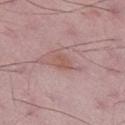<case>
<patient>
  <sex>male</sex>
  <age_approx>50</age_approx>
</patient>
<lesion_size>
  <long_diameter_mm_approx>2.5</long_diameter_mm_approx>
</lesion_size>
<site>right lower leg</site>
<image>
  <source>total-body photography crop</source>
  <field_of_view_mm>15</field_of_view_mm>
</image>
<automated_metrics>
  <eccentricity>0.7</eccentricity>
  <shape_asymmetry>0.25</shape_asymmetry>
  <border_irregularity_0_10>2.5</border_irregularity_0_10>
  <color_variation_0_10>1.5</color_variation_0_10>
  <peripheral_color_asymmetry>0.5</peripheral_color_asymmetry>
</automated_metrics>
<lighting>white-light</lighting>
</case>